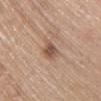{
  "biopsy_status": "not biopsied; imaged during a skin examination",
  "patient": {
    "sex": "female",
    "age_approx": 65
  },
  "site": "chest",
  "image": {
    "source": "total-body photography crop",
    "field_of_view_mm": 15
  }
}